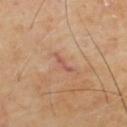| field | value |
|---|---|
| subject | male, about 65 years old |
| site | the upper back |
| TBP lesion metrics | an area of roughly 2.5 mm², a shape eccentricity near 0.95, and a symmetry-axis asymmetry near 0.45; a mean CIELAB color near L≈55 a*≈24 b*≈29; an automated nevus-likeness rating near 0 out of 100 |
| lighting | cross-polarized illumination |
| image | ~15 mm crop, total-body skin-cancer survey |